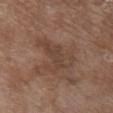Clinical impression: The lesion was tiled from a total-body skin photograph and was not biopsied. Context: Measured at roughly 5.5 mm in maximum diameter. The lesion is on the back. A female patient about 70 years old. A lesion tile, about 15 mm wide, cut from a 3D total-body photograph. The lesion-visualizer software estimated an average lesion color of about L≈42 a*≈17 b*≈25 (CIELAB), a lesion–skin lightness drop of about 6, and a normalized border contrast of about 5. The analysis additionally found a nevus-likeness score of about 0/100.